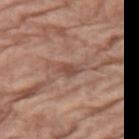Impression:
Recorded during total-body skin imaging; not selected for excision or biopsy.